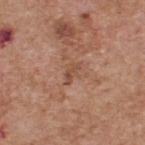Recorded during total-body skin imaging; not selected for excision or biopsy.